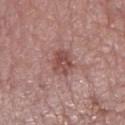The lesion was tiled from a total-body skin photograph and was not biopsied. The lesion is on the left lower leg. The patient is a female approximately 85 years of age or older. Measured at roughly 3 mm in maximum diameter. This image is a 15 mm lesion crop taken from a total-body photograph.